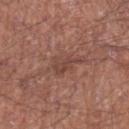Q: What are the patient's age and sex?
A: male, about 60 years old
Q: Lesion location?
A: the left forearm
Q: What lighting was used for the tile?
A: white-light
Q: How was this image acquired?
A: 15 mm crop, total-body photography